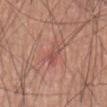<case>
  <biopsy_status>not biopsied; imaged during a skin examination</biopsy_status>
  <lighting>white-light</lighting>
  <patient>
    <sex>male</sex>
    <age_approx>60</age_approx>
  </patient>
  <automated_metrics>
    <area_mm2_approx>5.5</area_mm2_approx>
    <eccentricity>0.9</eccentricity>
    <shape_asymmetry>0.3</shape_asymmetry>
    <nevus_likeness_0_100>0</nevus_likeness_0_100>
  </automated_metrics>
  <image>
    <source>total-body photography crop</source>
    <field_of_view_mm>15</field_of_view_mm>
  </image>
  <lesion_size>
    <long_diameter_mm_approx>3.5</long_diameter_mm_approx>
  </lesion_size>
  <site>lower back</site>
</case>